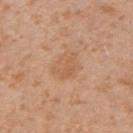| field | value |
|---|---|
| workup | no biopsy performed (imaged during a skin exam) |
| anatomic site | the right forearm |
| image source | ~15 mm tile from a whole-body skin photo |
| patient | female, approximately 30 years of age |